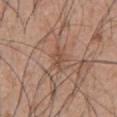Clinical impression: Captured during whole-body skin photography for melanoma surveillance; the lesion was not biopsied. Background: About 2.5 mm across. The tile uses white-light illumination. Located on the abdomen. A lesion tile, about 15 mm wide, cut from a 3D total-body photograph. A male patient aged approximately 55.